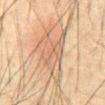Clinical impression: Recorded during total-body skin imaging; not selected for excision or biopsy. Image and clinical context: The total-body-photography lesion software estimated a footprint of about 22 mm², an outline eccentricity of about 0.75 (0 = round, 1 = elongated), and two-axis asymmetry of about 0.4. The analysis additionally found roughly 7 lightness units darker than nearby skin and a lesion-to-skin contrast of about 5 (normalized; higher = more distinct). From the abdomen. A male subject about 65 years old. This is a cross-polarized tile. A 15 mm close-up extracted from a 3D total-body photography capture.